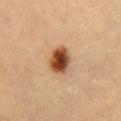{"biopsy_status": "not biopsied; imaged during a skin examination", "lesion_size": {"long_diameter_mm_approx": 3.5}, "lighting": "cross-polarized", "image": {"source": "total-body photography crop", "field_of_view_mm": 15}, "site": "lower back", "patient": {"sex": "female", "age_approx": 60}}